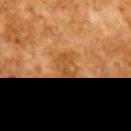biopsy status=total-body-photography surveillance lesion; no biopsy | patient=male, in their mid- to late 60s | tile lighting=cross-polarized illumination | image=total-body-photography crop, ~15 mm field of view.